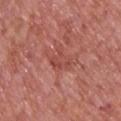Findings:
– notes — catalogued during a skin exam; not biopsied
– subject — male, approximately 75 years of age
– lesion diameter — ~3.5 mm (longest diameter)
– acquisition — ~15 mm tile from a whole-body skin photo
– automated lesion analysis — a normalized border contrast of about 5.5; an automated nevus-likeness rating near 0 out of 100
– anatomic site — the upper back